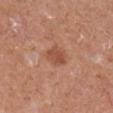follow-up = imaged on a skin check; not biopsied
imaging modality = total-body-photography crop, ~15 mm field of view
subject = male, aged 53–57
anatomic site = the right lower leg